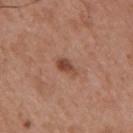* notes · catalogued during a skin exam; not biopsied
* subject · male, in their mid- to late 50s
* anatomic site · the mid back
* illumination · white-light
* image · total-body-photography crop, ~15 mm field of view